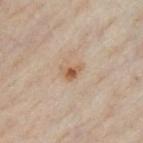Findings:
• follow-up: imaged on a skin check; not biopsied
• patient: male, approximately 50 years of age
• anatomic site: the front of the torso
• image: ~15 mm crop, total-body skin-cancer survey
• lesion diameter: ~3 mm (longest diameter)
• lighting: cross-polarized